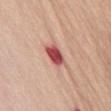Impression:
No biopsy was performed on this lesion — it was imaged during a full skin examination and was not determined to be concerning.
Clinical summary:
A female subject approximately 70 years of age. Measured at roughly 3 mm in maximum diameter. From the chest. An algorithmic analysis of the crop reported a lesion color around L≈51 a*≈35 b*≈25 in CIELAB, about 19 CIELAB-L* units darker than the surrounding skin, and a normalized border contrast of about 12.5. The software also gave a border-irregularity index near 1.5/10, a within-lesion color-variation index near 3.5/10, and peripheral color asymmetry of about 1. The tile uses white-light illumination. A region of skin cropped from a whole-body photographic capture, roughly 15 mm wide.